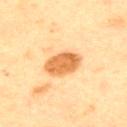Findings:
- follow-up — no biopsy performed (imaged during a skin exam)
- lesion diameter — ~4.5 mm (longest diameter)
- illumination — cross-polarized illumination
- image-analysis metrics — a footprint of about 11 mm², an outline eccentricity of about 0.75 (0 = round, 1 = elongated), and two-axis asymmetry of about 0.15; a lesion color around L≈55 a*≈21 b*≈39 in CIELAB, roughly 13 lightness units darker than nearby skin, and a normalized border contrast of about 9; a border-irregularity rating of about 1.5/10, internal color variation of about 3 on a 0–10 scale, and radial color variation of about 1; a classifier nevus-likeness of about 100/100
- subject — male, aged 63 to 67
- imaging modality — total-body-photography crop, ~15 mm field of view
- body site — the chest